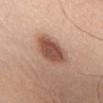Q: Is there a histopathology result?
A: imaged on a skin check; not biopsied
Q: Patient demographics?
A: male, aged 43–47
Q: How was the tile lit?
A: white-light
Q: Automated lesion metrics?
A: a lesion color around L≈52 a*≈22 b*≈28 in CIELAB, a lesion–skin lightness drop of about 15, and a normalized border contrast of about 10; a classifier nevus-likeness of about 100/100 and a lesion-detection confidence of about 100/100
Q: How was this image acquired?
A: ~15 mm crop, total-body skin-cancer survey
Q: Lesion location?
A: the chest
Q: Lesion size?
A: ≈5 mm